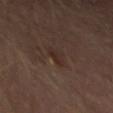Q: Was a biopsy performed?
A: no biopsy performed (imaged during a skin exam)
Q: What is the lesion's diameter?
A: ~3 mm (longest diameter)
Q: How was this image acquired?
A: ~15 mm crop, total-body skin-cancer survey
Q: Lesion location?
A: the left thigh
Q: Automated lesion metrics?
A: a mean CIELAB color near L≈26 a*≈14 b*≈20; internal color variation of about 2 on a 0–10 scale and a peripheral color-asymmetry measure near 0.5
Q: How was the tile lit?
A: cross-polarized
Q: Who is the patient?
A: male, aged 63–67